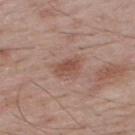<lesion>
  <biopsy_status>not biopsied; imaged during a skin examination</biopsy_status>
  <patient>
    <sex>male</sex>
    <age_approx>65</age_approx>
  </patient>
  <image>
    <source>total-body photography crop</source>
    <field_of_view_mm>15</field_of_view_mm>
  </image>
  <site>upper back</site>
  <lesion_size>
    <long_diameter_mm_approx>2.5</long_diameter_mm_approx>
  </lesion_size>
</lesion>